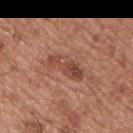Case summary:
* image source · 15 mm crop, total-body photography
* lighting · white-light
* size · about 4.5 mm
* location · the chest
* patient · male, in their mid-50s